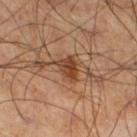Q: What is the lesion's diameter?
A: ~3.5 mm (longest diameter)
Q: Patient demographics?
A: male, aged 58–62
Q: What is the anatomic site?
A: the leg
Q: Illumination type?
A: cross-polarized
Q: What is the imaging modality?
A: ~15 mm tile from a whole-body skin photo